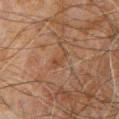<record>
  <biopsy_status>not biopsied; imaged during a skin examination</biopsy_status>
  <lighting>cross-polarized</lighting>
  <image>
    <source>total-body photography crop</source>
    <field_of_view_mm>15</field_of_view_mm>
  </image>
  <lesion_size>
    <long_diameter_mm_approx>2.5</long_diameter_mm_approx>
  </lesion_size>
  <patient>
    <sex>male</sex>
    <age_approx>60</age_approx>
  </patient>
  <site>chest</site>
</record>